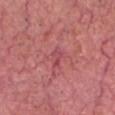Findings:
– follow-up: catalogued during a skin exam; not biopsied
– anatomic site: the head or neck
– subject: male, aged approximately 75
– image: total-body-photography crop, ~15 mm field of view